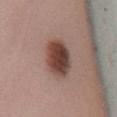Background:
The lesion is located on the right lower leg. About 4 mm across. The lesion-visualizer software estimated an area of roughly 12 mm² and an eccentricity of roughly 0.35. The software also gave a lesion color around L≈30 a*≈15 b*≈18 in CIELAB, roughly 12 lightness units darker than nearby skin, and a normalized lesion–skin contrast near 11.5. It also reported an automated nevus-likeness rating near 100 out of 100 and a detector confidence of about 100 out of 100 that the crop contains a lesion. The patient is a female aged around 20. A 15 mm close-up tile from a total-body photography series done for melanoma screening. Captured under cross-polarized illumination.